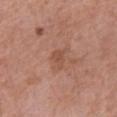The lesion was photographed on a routine skin check and not biopsied; there is no pathology result.
This image is a 15 mm lesion crop taken from a total-body photograph.
From the right forearm.
The subject is a female in their mid-50s.
Automated tile analysis of the lesion measured a lesion area of about 3 mm², an outline eccentricity of about 0.85 (0 = round, 1 = elongated), and a shape-asymmetry score of about 0.4 (0 = symmetric). The software also gave an average lesion color of about L≈50 a*≈23 b*≈29 (CIELAB), a lesion–skin lightness drop of about 7, and a normalized border contrast of about 5.5. And it measured border irregularity of about 3.5 on a 0–10 scale, internal color variation of about 0.5 on a 0–10 scale, and a peripheral color-asymmetry measure near 0. The software also gave a lesion-detection confidence of about 100/100.
The recorded lesion diameter is about 2.5 mm.